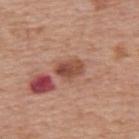biopsy_status: not biopsied; imaged during a skin examination
image:
  source: total-body photography crop
  field_of_view_mm: 15
lighting: white-light
lesion_size:
  long_diameter_mm_approx: 3.5
patient:
  sex: male
  age_approx: 75
site: upper back